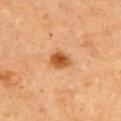Assessment: No biopsy was performed on this lesion — it was imaged during a full skin examination and was not determined to be concerning. Context: The lesion is on the upper back. Cropped from a whole-body photographic skin survey; the tile spans about 15 mm. Imaged with cross-polarized lighting. Measured at roughly 2.5 mm in maximum diameter. A male subject, about 55 years old.